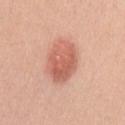  biopsy_status: not biopsied; imaged during a skin examination
  patient:
    sex: female
    age_approx: 35
  lighting: white-light
  image:
    source: total-body photography crop
    field_of_view_mm: 15
  automated_metrics:
    area_mm2_approx: 16.0
    eccentricity: 0.6
    shape_asymmetry: 0.1
    cielab_L: 61
    cielab_a: 27
    cielab_b: 31
    vs_skin_darker_L: 11.0
    vs_skin_contrast_norm: 7.0
    nevus_likeness_0_100: 100
    lesion_detection_confidence_0_100: 100
  lesion_size:
    long_diameter_mm_approx: 5.0
  site: left upper arm